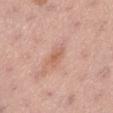Q: Was this lesion biopsied?
A: total-body-photography surveillance lesion; no biopsy
Q: Patient demographics?
A: female, roughly 55 years of age
Q: How was this image acquired?
A: 15 mm crop, total-body photography
Q: How was the tile lit?
A: white-light illumination
Q: What is the anatomic site?
A: the left lower leg